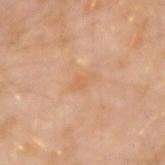workup: imaged on a skin check; not biopsied | image: total-body-photography crop, ~15 mm field of view | body site: the arm | lesion diameter: ~2.5 mm (longest diameter) | subject: male, about 30 years old.